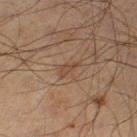Q: Was this lesion biopsied?
A: no biopsy performed (imaged during a skin exam)
Q: How was the tile lit?
A: cross-polarized
Q: Where on the body is the lesion?
A: the left thigh
Q: How was this image acquired?
A: ~15 mm tile from a whole-body skin photo
Q: Automated lesion metrics?
A: an area of roughly 2.5 mm², an eccentricity of roughly 0.9, and a shape-asymmetry score of about 0.55 (0 = symmetric); a lesion color around L≈36 a*≈15 b*≈25 in CIELAB, a lesion–skin lightness drop of about 6, and a lesion-to-skin contrast of about 5.5 (normalized; higher = more distinct); a nevus-likeness score of about 0/100 and a lesion-detection confidence of about 100/100
Q: How large is the lesion?
A: ≈2.5 mm
Q: Who is the patient?
A: male, aged 68–72